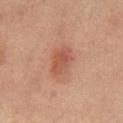| feature | finding |
|---|---|
| notes | total-body-photography surveillance lesion; no biopsy |
| site | the mid back |
| subject | male, roughly 65 years of age |
| image | ~15 mm crop, total-body skin-cancer survey |